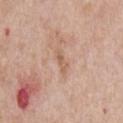Q: Is there a histopathology result?
A: no biopsy performed (imaged during a skin exam)
Q: What is the anatomic site?
A: the chest
Q: What is the imaging modality?
A: ~15 mm tile from a whole-body skin photo
Q: What lighting was used for the tile?
A: white-light
Q: What did automated image analysis measure?
A: an area of roughly 2 mm² and a symmetry-axis asymmetry near 0.45; roughly 8 lightness units darker than nearby skin
Q: Patient demographics?
A: male, roughly 60 years of age